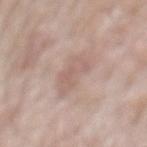This lesion was catalogued during total-body skin photography and was not selected for biopsy.
On the mid back.
The recorded lesion diameter is about 4.5 mm.
Imaged with white-light lighting.
Cropped from a total-body skin-imaging series; the visible field is about 15 mm.
A male patient, about 55 years old.